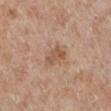No biopsy was performed on this lesion — it was imaged during a full skin examination and was not determined to be concerning. The lesion's longest dimension is about 3 mm. The patient is a female about 60 years old. The lesion is located on the right lower leg. A region of skin cropped from a whole-body photographic capture, roughly 15 mm wide. This is a white-light tile.